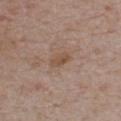Clinical impression: This lesion was catalogued during total-body skin photography and was not selected for biopsy. Context: The lesion's longest dimension is about 2.5 mm. Imaged with white-light lighting. The patient is a male approximately 75 years of age. On the chest. Cropped from a total-body skin-imaging series; the visible field is about 15 mm.